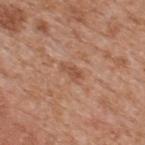follow-up: total-body-photography surveillance lesion; no biopsy
TBP lesion metrics: a border-irregularity index near 3.5/10, a color-variation rating of about 1/10, and a peripheral color-asymmetry measure near 0.5; an automated nevus-likeness rating near 0 out of 100 and a detector confidence of about 100 out of 100 that the crop contains a lesion
patient: male, about 65 years old
lesion size: ~2.5 mm (longest diameter)
image: ~15 mm crop, total-body skin-cancer survey
anatomic site: the upper back
lighting: white-light